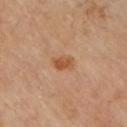Clinical impression: The lesion was photographed on a routine skin check and not biopsied; there is no pathology result.